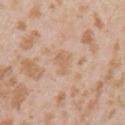Recorded during total-body skin imaging; not selected for excision or biopsy.
On the left upper arm.
A female patient approximately 25 years of age.
Imaged with white-light lighting.
An algorithmic analysis of the crop reported border irregularity of about 4 on a 0–10 scale, a color-variation rating of about 0.5/10, and radial color variation of about 0.
A roughly 15 mm field-of-view crop from a total-body skin photograph.